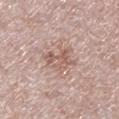No biopsy was performed on this lesion — it was imaged during a full skin examination and was not determined to be concerning. A male patient about 65 years old. From the right lower leg. A lesion tile, about 15 mm wide, cut from a 3D total-body photograph.